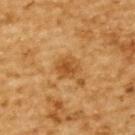The lesion was tiled from a total-body skin photograph and was not biopsied. Cropped from a total-body skin-imaging series; the visible field is about 15 mm. The recorded lesion diameter is about 2.5 mm. From the upper back. A male subject aged 83 or over.